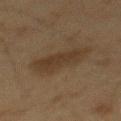• workup · no biopsy performed (imaged during a skin exam)
• imaging modality · ~15 mm crop, total-body skin-cancer survey
• location · the mid back
• subject · male, aged approximately 60
• automated metrics · a footprint of about 16 mm², an outline eccentricity of about 0.9 (0 = round, 1 = elongated), and a shape-asymmetry score of about 0.25 (0 = symmetric); an automated nevus-likeness rating near 60 out of 100 and a lesion-detection confidence of about 100/100
• diameter · ≈7 mm